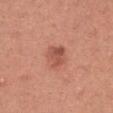notes: total-body-photography surveillance lesion; no biopsy | subject: female, aged approximately 20 | image source: ~15 mm crop, total-body skin-cancer survey | image-analysis metrics: an area of roughly 6.5 mm² and two-axis asymmetry of about 0.25; a lesion color around L≈53 a*≈27 b*≈30 in CIELAB, roughly 10 lightness units darker than nearby skin, and a normalized border contrast of about 6.5; border irregularity of about 2.5 on a 0–10 scale, internal color variation of about 4 on a 0–10 scale, and radial color variation of about 1.5; an automated nevus-likeness rating near 55 out of 100 and a lesion-detection confidence of about 100/100 | site: the right upper arm | lesion diameter: ~3 mm (longest diameter).